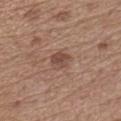Captured under white-light illumination. Automated image analysis of the tile measured a lesion area of about 5 mm² and a shape-asymmetry score of about 0.25 (0 = symmetric). The analysis additionally found an average lesion color of about L≈47 a*≈19 b*≈25 (CIELAB), about 9 CIELAB-L* units darker than the surrounding skin, and a lesion-to-skin contrast of about 7 (normalized; higher = more distinct). The subject is a male roughly 65 years of age. The lesion's longest dimension is about 2.5 mm. Located on the front of the torso. This image is a 15 mm lesion crop taken from a total-body photograph.